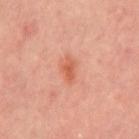Notes:
- workup — imaged on a skin check; not biopsied
- site — the mid back
- automated lesion analysis — an area of roughly 3.5 mm², a shape eccentricity near 0.8, and a shape-asymmetry score of about 0.3 (0 = symmetric); roughly 9 lightness units darker than nearby skin; radial color variation of about 0.5
- image source — ~15 mm tile from a whole-body skin photo
- lesion diameter — about 2.5 mm
- subject — male, in their mid- to late 40s